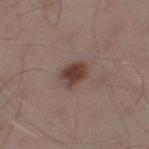{
  "biopsy_status": "not biopsied; imaged during a skin examination",
  "patient": {
    "sex": "male",
    "age_approx": 55
  },
  "image": {
    "source": "total-body photography crop",
    "field_of_view_mm": 15
  },
  "site": "leg"
}